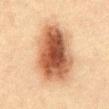Case summary:
* follow-up — catalogued during a skin exam; not biopsied
* tile lighting — cross-polarized illumination
* automated metrics — a classifier nevus-likeness of about 100/100 and a lesion-detection confidence of about 100/100
* patient — male, aged 48–52
* location — the abdomen
* lesion diameter — about 7.5 mm
* imaging modality — total-body-photography crop, ~15 mm field of view